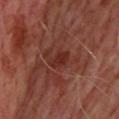biopsy status: total-body-photography surveillance lesion; no biopsy | diameter: about 3 mm | patient: male, roughly 65 years of age | site: the upper back | lighting: cross-polarized | imaging modality: 15 mm crop, total-body photography | automated lesion analysis: a footprint of about 4 mm², an eccentricity of roughly 0.75, and a shape-asymmetry score of about 0.25 (0 = symmetric); a mean CIELAB color near L≈28 a*≈26 b*≈25 and a lesion–skin lightness drop of about 6; a color-variation rating of about 1/10 and a peripheral color-asymmetry measure near 0.5.